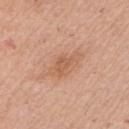Assessment: The lesion was photographed on a routine skin check and not biopsied; there is no pathology result. Background: The lesion is located on the arm. A female subject, aged around 65. Longest diameter approximately 4 mm. This image is a 15 mm lesion crop taken from a total-body photograph.